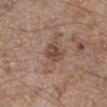notes = imaged on a skin check; not biopsied | lighting = white-light | size = about 2.5 mm | image source = total-body-photography crop, ~15 mm field of view | anatomic site = the right lower leg | TBP lesion metrics = a shape eccentricity near 0.65 and a symmetry-axis asymmetry near 0.25; a lesion color around L≈45 a*≈18 b*≈26 in CIELAB, about 9 CIELAB-L* units darker than the surrounding skin, and a normalized border contrast of about 7; border irregularity of about 2 on a 0–10 scale and internal color variation of about 3 on a 0–10 scale; a classifier nevus-likeness of about 0/100 and a detector confidence of about 100 out of 100 that the crop contains a lesion | patient = male, about 65 years old.